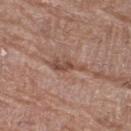Context: The lesion-visualizer software estimated a footprint of about 5 mm² and a shape-asymmetry score of about 0.5 (0 = symmetric). It also reported a lesion color around L≈48 a*≈20 b*≈27 in CIELAB, a lesion–skin lightness drop of about 10, and a normalized border contrast of about 7. The software also gave a lesion-detection confidence of about 85/100. A region of skin cropped from a whole-body photographic capture, roughly 15 mm wide. Measured at roughly 4 mm in maximum diameter. A female patient approximately 80 years of age. From the right thigh.